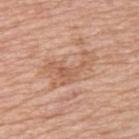Impression:
Captured during whole-body skin photography for melanoma surveillance; the lesion was not biopsied.
Clinical summary:
This image is a 15 mm lesion crop taken from a total-body photograph. A female patient roughly 45 years of age. Automated tile analysis of the lesion measured an average lesion color of about L≈60 a*≈21 b*≈32 (CIELAB) and roughly 8 lightness units darker than nearby skin. It also reported a peripheral color-asymmetry measure near 1. Located on the upper back.